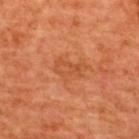Q: Was this lesion biopsied?
A: total-body-photography surveillance lesion; no biopsy
Q: Automated lesion metrics?
A: an outline eccentricity of about 0.85 (0 = round, 1 = elongated) and a symmetry-axis asymmetry near 0.4; roughly 6 lightness units darker than nearby skin and a normalized lesion–skin contrast near 5; a border-irregularity index near 4.5/10, a within-lesion color-variation index near 3/10, and peripheral color asymmetry of about 1; a nevus-likeness score of about 10/100 and lesion-presence confidence of about 100/100
Q: How large is the lesion?
A: ≈4 mm
Q: What is the imaging modality?
A: 15 mm crop, total-body photography
Q: Lesion location?
A: the upper back
Q: What are the patient's age and sex?
A: male, aged approximately 65
Q: How was the tile lit?
A: cross-polarized illumination